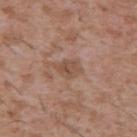Imaged during a routine full-body skin examination; the lesion was not biopsied and no histopathology is available.
A male subject, aged 43 to 47.
The tile uses white-light illumination.
A close-up tile cropped from a whole-body skin photograph, about 15 mm across.
The lesion is on the upper back.
The recorded lesion diameter is about 3 mm.